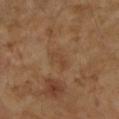Clinical impression:
This lesion was catalogued during total-body skin photography and was not selected for biopsy.
Acquisition and patient details:
A 15 mm close-up extracted from a 3D total-body photography capture. The lesion is on the left forearm. A female patient, aged around 70. Captured under cross-polarized illumination. Longest diameter approximately 2.5 mm.